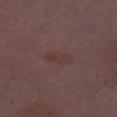| key | value |
|---|---|
| body site | the left lower leg |
| imaging modality | total-body-photography crop, ~15 mm field of view |
| diameter | about 4 mm |
| subject | female, roughly 30 years of age |
| tile lighting | white-light illumination |